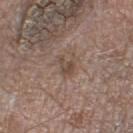Image and clinical context: Automated image analysis of the tile measured a classifier nevus-likeness of about 0/100 and a lesion-detection confidence of about 100/100. Measured at roughly 2.5 mm in maximum diameter. A lesion tile, about 15 mm wide, cut from a 3D total-body photograph. A male subject roughly 75 years of age. On the left thigh. Captured under white-light illumination.